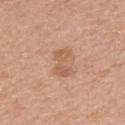Clinical impression:
Imaged during a routine full-body skin examination; the lesion was not biopsied and no histopathology is available.
Image and clinical context:
Longest diameter approximately 4 mm. A 15 mm close-up extracted from a 3D total-body photography capture. Located on the right forearm. This is a white-light tile. An algorithmic analysis of the crop reported an area of roughly 6 mm², an outline eccentricity of about 0.9 (0 = round, 1 = elongated), and a symmetry-axis asymmetry near 0.35. The analysis additionally found a lesion color around L≈59 a*≈22 b*≈32 in CIELAB, about 8 CIELAB-L* units darker than the surrounding skin, and a normalized lesion–skin contrast near 5.5. And it measured a border-irregularity rating of about 5/10, a color-variation rating of about 2.5/10, and peripheral color asymmetry of about 1. A female patient aged 33–37.